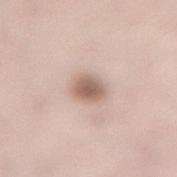Recorded during total-body skin imaging; not selected for excision or biopsy. The lesion is located on the lower back. A close-up tile cropped from a whole-body skin photograph, about 15 mm across. A female subject, about 50 years old. The lesion's longest dimension is about 3 mm.